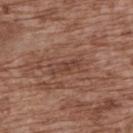Clinical impression:
Recorded during total-body skin imaging; not selected for excision or biopsy.
Clinical summary:
This image is a 15 mm lesion crop taken from a total-body photograph. The tile uses white-light illumination. The lesion is located on the upper back. An algorithmic analysis of the crop reported an area of roughly 3.5 mm² and an eccentricity of roughly 0.95. The patient is a female aged 73 to 77.